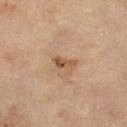Clinical impression:
The lesion was photographed on a routine skin check and not biopsied; there is no pathology result.
Background:
A female patient approximately 70 years of age. A 15 mm close-up tile from a total-body photography series done for melanoma screening. The lesion is located on the left lower leg.